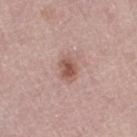Recorded during total-body skin imaging; not selected for excision or biopsy.
This is a white-light tile.
From the right thigh.
The patient is a female aged approximately 50.
A 15 mm crop from a total-body photograph taken for skin-cancer surveillance.
Measured at roughly 3 mm in maximum diameter.